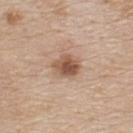notes=imaged on a skin check; not biopsied
patient=male, aged 78 to 82
acquisition=total-body-photography crop, ~15 mm field of view
anatomic site=the upper back